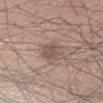This lesion was catalogued during total-body skin photography and was not selected for biopsy. A male subject, in their mid- to late 50s. A lesion tile, about 15 mm wide, cut from a 3D total-body photograph.